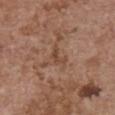Clinical impression: Imaged during a routine full-body skin examination; the lesion was not biopsied and no histopathology is available. Image and clinical context: A 15 mm crop from a total-body photograph taken for skin-cancer surveillance. The lesion is located on the chest. The tile uses white-light illumination. The lesion's longest dimension is about 2.5 mm. A female patient, approximately 65 years of age.